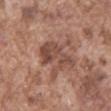A male subject aged 73 to 77. A close-up tile cropped from a whole-body skin photograph, about 15 mm across. The lesion is located on the abdomen.